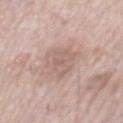This lesion was catalogued during total-body skin photography and was not selected for biopsy. The lesion is on the mid back. A 15 mm close-up extracted from a 3D total-body photography capture. Measured at roughly 5 mm in maximum diameter. The patient is a male about 75 years old.